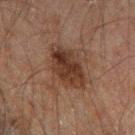<case>
  <automated_metrics>
    <cielab_L>27</cielab_L>
    <cielab_a>15</cielab_a>
    <cielab_b>21</cielab_b>
    <vs_skin_darker_L>9.0</vs_skin_darker_L>
    <vs_skin_contrast_norm>9.5</vs_skin_contrast_norm>
    <nevus_likeness_0_100>30</nevus_likeness_0_100>
    <lesion_detection_confidence_0_100>100</lesion_detection_confidence_0_100>
  </automated_metrics>
  <lighting>cross-polarized</lighting>
  <site>left thigh</site>
  <image>
    <source>total-body photography crop</source>
    <field_of_view_mm>15</field_of_view_mm>
  </image>
  <lesion_size>
    <long_diameter_mm_approx>6.5</long_diameter_mm_approx>
  </lesion_size>
  <patient>
    <sex>male</sex>
    <age_approx>60</age_approx>
  </patient>
</case>